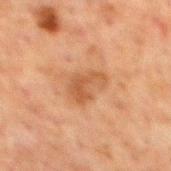Captured during whole-body skin photography for melanoma surveillance; the lesion was not biopsied. This is a cross-polarized tile. An algorithmic analysis of the crop reported a footprint of about 7 mm². And it measured a lesion color around L≈42 a*≈19 b*≈31 in CIELAB, roughly 7 lightness units darker than nearby skin, and a normalized lesion–skin contrast near 6.5. And it measured an automated nevus-likeness rating near 0 out of 100. About 4 mm across. From the mid back. The subject is a male roughly 60 years of age. Cropped from a total-body skin-imaging series; the visible field is about 15 mm.